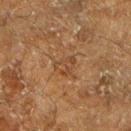Image and clinical context: The patient is a male in their 60s. The tile uses cross-polarized illumination. A region of skin cropped from a whole-body photographic capture, roughly 15 mm wide. From the left lower leg.